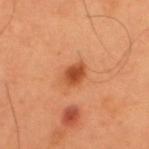| feature | finding |
|---|---|
| workup | no biopsy performed (imaged during a skin exam) |
| size | about 3 mm |
| image | total-body-photography crop, ~15 mm field of view |
| patient | male, roughly 55 years of age |
| body site | the upper back |